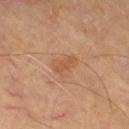No biopsy was performed on this lesion — it was imaged during a full skin examination and was not determined to be concerning.
A subject in their mid- to late 60s.
The lesion is on the leg.
A close-up tile cropped from a whole-body skin photograph, about 15 mm across.